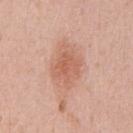<case>
<biopsy_status>not biopsied; imaged during a skin examination</biopsy_status>
<automated_metrics>
  <area_mm2_approx>12.0</area_mm2_approx>
  <eccentricity>0.7</eccentricity>
  <nevus_likeness_0_100>45</nevus_likeness_0_100>
</automated_metrics>
<patient>
  <sex>male</sex>
  <age_approx>55</age_approx>
</patient>
<image>
  <source>total-body photography crop</source>
  <field_of_view_mm>15</field_of_view_mm>
</image>
<site>chest</site>
</case>